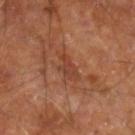workup: imaged on a skin check; not biopsied | patient: male, aged approximately 60 | image: 15 mm crop, total-body photography | location: the right leg.